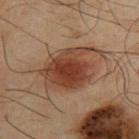  biopsy_status: not biopsied; imaged during a skin examination
  patient:
    sex: male
    age_approx: 65
  site: upper back
  image:
    source: total-body photography crop
    field_of_view_mm: 15